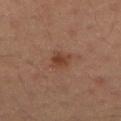The lesion was tiled from a total-body skin photograph and was not biopsied.
The lesion-visualizer software estimated a footprint of about 5 mm² and a shape-asymmetry score of about 0.25 (0 = symmetric). The analysis additionally found a mean CIELAB color near L≈29 a*≈17 b*≈22, roughly 6 lightness units darker than nearby skin, and a normalized lesion–skin contrast near 7. The software also gave a classifier nevus-likeness of about 40/100 and lesion-presence confidence of about 100/100.
Captured under cross-polarized illumination.
A male subject in their 50s.
The lesion's longest dimension is about 2.5 mm.
A 15 mm crop from a total-body photograph taken for skin-cancer surveillance.
On the abdomen.